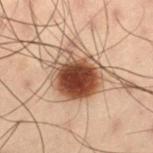This lesion was catalogued during total-body skin photography and was not selected for biopsy. Approximately 5.5 mm at its widest. From the left thigh. Captured under cross-polarized illumination. Automated image analysis of the tile measured lesion-presence confidence of about 100/100. A region of skin cropped from a whole-body photographic capture, roughly 15 mm wide. A male subject, aged 53–57.